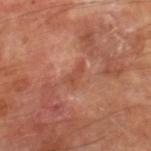Clinical impression:
This lesion was catalogued during total-body skin photography and was not selected for biopsy.
Context:
The total-body-photography lesion software estimated a lesion–skin lightness drop of about 7 and a normalized border contrast of about 5. The software also gave an automated nevus-likeness rating near 0 out of 100 and a lesion-detection confidence of about 100/100. From the right lower leg. Captured under cross-polarized illumination. This image is a 15 mm lesion crop taken from a total-body photograph. A male subject, aged 68 to 72.